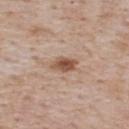follow-up = catalogued during a skin exam; not biopsied
lesion diameter = about 3.5 mm
subject = male, approximately 75 years of age
body site = the upper back
imaging modality = total-body-photography crop, ~15 mm field of view